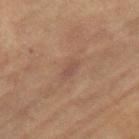Case summary:
• notes · catalogued during a skin exam; not biopsied
• TBP lesion metrics · a shape eccentricity near 0.9 and a symmetry-axis asymmetry near 0.3; a normalized lesion–skin contrast near 5.5; a border-irregularity rating of about 3/10, a color-variation rating of about 1.5/10, and a peripheral color-asymmetry measure near 0.5; a lesion-detection confidence of about 95/100
• location · the right thigh
• image · ~15 mm crop, total-body skin-cancer survey
• patient · female, about 70 years old